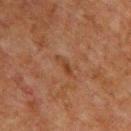Recorded during total-body skin imaging; not selected for excision or biopsy.
This is a cross-polarized tile.
Automated tile analysis of the lesion measured a border-irregularity rating of about 4.5/10 and a color-variation rating of about 0/10. It also reported a classifier nevus-likeness of about 0/100 and lesion-presence confidence of about 95/100.
A roughly 15 mm field-of-view crop from a total-body skin photograph.
A male patient, in their 60s.
The lesion's longest dimension is about 3 mm.
Located on the upper back.